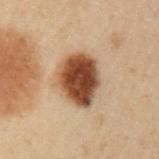Assessment: This lesion was catalogued during total-body skin photography and was not selected for biopsy. Background: Located on the arm. A male subject, about 55 years old. Imaged with cross-polarized lighting. A 15 mm close-up tile from a total-body photography series done for melanoma screening. The lesion-visualizer software estimated an area of roughly 17 mm², an outline eccentricity of about 0.6 (0 = round, 1 = elongated), and a shape-asymmetry score of about 0.2 (0 = symmetric). It also reported roughly 18 lightness units darker than nearby skin and a lesion-to-skin contrast of about 14.5 (normalized; higher = more distinct). The analysis additionally found border irregularity of about 2 on a 0–10 scale, internal color variation of about 5.5 on a 0–10 scale, and peripheral color asymmetry of about 2. And it measured a lesion-detection confidence of about 100/100. The lesion's longest dimension is about 5 mm.